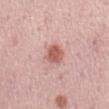Automated tile analysis of the lesion measured an area of roughly 5.5 mm² and a symmetry-axis asymmetry near 0.2. The patient is a male aged approximately 60. Imaged with white-light lighting. On the mid back. About 3 mm across. A 15 mm crop from a total-body photograph taken for skin-cancer surveillance.